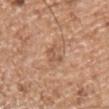Clinical impression:
Recorded during total-body skin imaging; not selected for excision or biopsy.
Acquisition and patient details:
A male patient aged approximately 55. Located on the left upper arm. This image is a 15 mm lesion crop taken from a total-body photograph. Longest diameter approximately 2.5 mm. The tile uses white-light illumination.